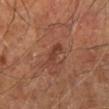The lesion was photographed on a routine skin check and not biopsied; there is no pathology result.
A region of skin cropped from a whole-body photographic capture, roughly 15 mm wide.
The subject is a male aged 58–62.
From the right leg.
About 3.5 mm across.
This is a cross-polarized tile.
An algorithmic analysis of the crop reported a footprint of about 4 mm², a shape eccentricity near 0.9, and a shape-asymmetry score of about 0.35 (0 = symmetric). And it measured a lesion color around L≈38 a*≈22 b*≈27 in CIELAB, about 7 CIELAB-L* units darker than the surrounding skin, and a normalized lesion–skin contrast near 6.